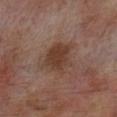Q: Is there a histopathology result?
A: total-body-photography surveillance lesion; no biopsy
Q: What kind of image is this?
A: 15 mm crop, total-body photography
Q: Who is the patient?
A: male, about 70 years old
Q: Where on the body is the lesion?
A: the leg
Q: Lesion size?
A: about 4.5 mm